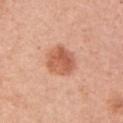Assessment:
Part of a total-body skin-imaging series; this lesion was reviewed on a skin check and was not flagged for biopsy.
Context:
A 15 mm close-up tile from a total-body photography series done for melanoma screening. A female patient, aged approximately 60. From the left upper arm.